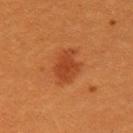Recorded during total-body skin imaging; not selected for excision or biopsy. The lesion-visualizer software estimated a lesion area of about 10 mm², an eccentricity of roughly 0.75, and a shape-asymmetry score of about 0.2 (0 = symmetric). It also reported a lesion color around L≈42 a*≈29 b*≈39 in CIELAB, roughly 8 lightness units darker than nearby skin, and a normalized lesion–skin contrast near 6.5. The analysis additionally found a border-irregularity index near 3/10, internal color variation of about 2.5 on a 0–10 scale, and radial color variation of about 1. A 15 mm crop from a total-body photograph taken for skin-cancer surveillance. A female subject aged 28–32. Approximately 4.5 mm at its widest. Located on the arm. Imaged with cross-polarized lighting.